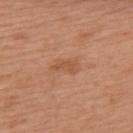{"biopsy_status": "not biopsied; imaged during a skin examination", "image": {"source": "total-body photography crop", "field_of_view_mm": 15}, "lesion_size": {"long_diameter_mm_approx": 3.5}, "site": "upper back", "patient": {"sex": "female", "age_approx": 40}, "lighting": "white-light"}